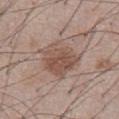The lesion was tiled from a total-body skin photograph and was not biopsied. A male patient aged 53–57. This is a white-light tile. This image is a 15 mm lesion crop taken from a total-body photograph. Located on the front of the torso.